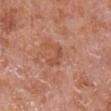notes: total-body-photography surveillance lesion; no biopsy
image: ~15 mm tile from a whole-body skin photo
patient: male, aged approximately 65
illumination: white-light
body site: the arm